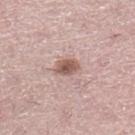Assessment: Part of a total-body skin-imaging series; this lesion was reviewed on a skin check and was not flagged for biopsy. Image and clinical context: The patient is a female about 40 years old. The lesion is on the right lower leg. A 15 mm crop from a total-body photograph taken for skin-cancer surveillance.